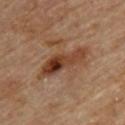Part of a total-body skin-imaging series; this lesion was reviewed on a skin check and was not flagged for biopsy. This is a cross-polarized tile. The lesion-visualizer software estimated an area of roughly 12 mm², an eccentricity of roughly 0.95, and a symmetry-axis asymmetry near 0.35. The software also gave roughly 12 lightness units darker than nearby skin and a lesion-to-skin contrast of about 9.5 (normalized; higher = more distinct). Cropped from a whole-body photographic skin survey; the tile spans about 15 mm. The subject is a male aged 83 or older. On the back.